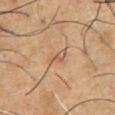Part of a total-body skin-imaging series; this lesion was reviewed on a skin check and was not flagged for biopsy. Measured at roughly 2.5 mm in maximum diameter. The patient is a male aged 53–57. The lesion-visualizer software estimated an area of roughly 3.5 mm² and an outline eccentricity of about 0.8 (0 = round, 1 = elongated). The software also gave an average lesion color of about L≈56 a*≈18 b*≈32 (CIELAB) and a normalized lesion–skin contrast near 5.5. The analysis additionally found border irregularity of about 3 on a 0–10 scale and a peripheral color-asymmetry measure near 1.5. A 15 mm close-up tile from a total-body photography series done for melanoma screening. From the chest.